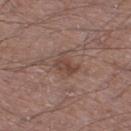workup: imaged on a skin check; not biopsied.